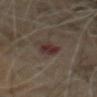follow-up: total-body-photography surveillance lesion; no biopsy | lesion size: ~3.5 mm (longest diameter) | subject: male, aged 58–62 | acquisition: ~15 mm crop, total-body skin-cancer survey | body site: the mid back | illumination: cross-polarized | automated metrics: a footprint of about 5 mm², an eccentricity of roughly 0.8, and two-axis asymmetry of about 0.3; roughly 8 lightness units darker than nearby skin and a normalized border contrast of about 9; a border-irregularity index near 3/10 and peripheral color asymmetry of about 1.5; a classifier nevus-likeness of about 0/100.